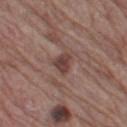{"biopsy_status": "not biopsied; imaged during a skin examination", "lesion_size": {"long_diameter_mm_approx": 2.5}, "lighting": "white-light", "site": "left thigh", "image": {"source": "total-body photography crop", "field_of_view_mm": 15}, "patient": {"sex": "male", "age_approx": 65}, "automated_metrics": {"border_irregularity_0_10": 3.0, "peripheral_color_asymmetry": 1.0, "nevus_likeness_0_100": 45, "lesion_detection_confidence_0_100": 100}}